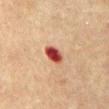Part of a total-body skin-imaging series; this lesion was reviewed on a skin check and was not flagged for biopsy. The lesion's longest dimension is about 3 mm. A male patient aged 73–77. Cropped from a total-body skin-imaging series; the visible field is about 15 mm. The total-body-photography lesion software estimated a footprint of about 4.5 mm², a shape eccentricity near 0.7, and a symmetry-axis asymmetry near 0.15. The software also gave border irregularity of about 1.5 on a 0–10 scale, a within-lesion color-variation index near 3/10, and radial color variation of about 1. And it measured an automated nevus-likeness rating near 0 out of 100. On the abdomen.